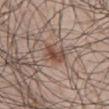<tbp_lesion>
<biopsy_status>not biopsied; imaged during a skin examination</biopsy_status>
<lighting>white-light</lighting>
<patient>
  <sex>male</sex>
  <age_approx>50</age_approx>
</patient>
<image>
  <source>total-body photography crop</source>
  <field_of_view_mm>15</field_of_view_mm>
</image>
<site>abdomen</site>
<lesion_size>
  <long_diameter_mm_approx>3.0</long_diameter_mm_approx>
</lesion_size>
<automated_metrics>
  <cielab_L>48</cielab_L>
  <cielab_a>18</cielab_a>
  <cielab_b>25</cielab_b>
  <vs_skin_darker_L>10.0</vs_skin_darker_L>
  <vs_skin_contrast_norm>7.5</vs_skin_contrast_norm>
  <nevus_likeness_0_100>95</nevus_likeness_0_100>
  <lesion_detection_confidence_0_100>100</lesion_detection_confidence_0_100>
</automated_metrics>
</tbp_lesion>